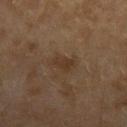{"biopsy_status": "not biopsied; imaged during a skin examination", "lesion_size": {"long_diameter_mm_approx": 2.5}, "lighting": "cross-polarized", "image": {"source": "total-body photography crop", "field_of_view_mm": 15}, "patient": {"sex": "female", "age_approx": 60}, "site": "left forearm"}